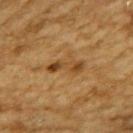The lesion was tiled from a total-body skin photograph and was not biopsied.
The patient is a male aged around 85 or older.
A close-up tile cropped from a whole-body skin photograph, about 15 mm across.
From the upper back.
The tile uses cross-polarized illumination.
The lesion's longest dimension is about 4.5 mm.